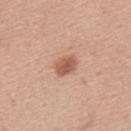Findings:
– notes: imaged on a skin check; not biopsied
– acquisition: ~15 mm crop, total-body skin-cancer survey
– diameter: ≈3 mm
– illumination: white-light
– location: the upper back
– patient: male, aged 23 to 27